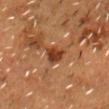Impression:
No biopsy was performed on this lesion — it was imaged during a full skin examination and was not determined to be concerning.
Image and clinical context:
The subject is a male approximately 55 years of age. A region of skin cropped from a whole-body photographic capture, roughly 15 mm wide. About 3 mm across. From the head or neck.